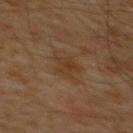location: the mid back; imaging modality: ~15 mm crop, total-body skin-cancer survey; lesion diameter: about 3.5 mm; subject: male, roughly 60 years of age; tile lighting: cross-polarized illumination.